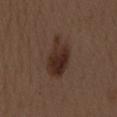<record>
  <biopsy_status>not biopsied; imaged during a skin examination</biopsy_status>
  <site>back</site>
  <automated_metrics>
    <area_mm2_approx>14.0</area_mm2_approx>
    <eccentricity>0.9</eccentricity>
    <shape_asymmetry>0.25</shape_asymmetry>
    <cielab_L>29</cielab_L>
    <cielab_a>16</cielab_a>
    <cielab_b>22</cielab_b>
    <vs_skin_darker_L>9.0</vs_skin_darker_L>
    <vs_skin_contrast_norm>9.5</vs_skin_contrast_norm>
    <border_irregularity_0_10>3.5</border_irregularity_0_10>
    <color_variation_0_10>5.5</color_variation_0_10>
    <peripheral_color_asymmetry>2.0</peripheral_color_asymmetry>
  </automated_metrics>
  <lesion_size>
    <long_diameter_mm_approx>6.0</long_diameter_mm_approx>
  </lesion_size>
  <image>
    <source>total-body photography crop</source>
    <field_of_view_mm>15</field_of_view_mm>
  </image>
  <lighting>white-light</lighting>
  <patient>
    <sex>female</sex>
    <age_approx>50</age_approx>
  </patient>
</record>